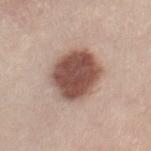workup: imaged on a skin check; not biopsied | lesion diameter: ≈6 mm | subject: female, aged 43 to 47 | acquisition: ~15 mm tile from a whole-body skin photo | anatomic site: the chest | automated lesion analysis: a lesion area of about 22 mm²; a lesion–skin lightness drop of about 18 and a normalized lesion–skin contrast near 12.